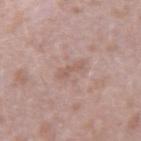Notes:
- subject: male, aged 38–42
- imaging modality: total-body-photography crop, ~15 mm field of view
- anatomic site: the right upper arm